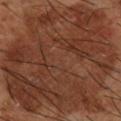{"biopsy_status": "not biopsied; imaged during a skin examination", "lighting": "cross-polarized", "lesion_size": {"long_diameter_mm_approx": 14.5}, "patient": {"sex": "male", "age_approx": 65}, "site": "right lower leg", "image": {"source": "total-body photography crop", "field_of_view_mm": 15}}